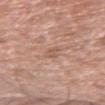biopsy status = total-body-photography surveillance lesion; no biopsy
imaging modality = total-body-photography crop, ~15 mm field of view
lesion diameter = ≈3 mm
patient = male, roughly 80 years of age
anatomic site = the left upper arm
illumination = white-light